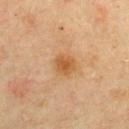Assessment: Part of a total-body skin-imaging series; this lesion was reviewed on a skin check and was not flagged for biopsy. Background: The total-body-photography lesion software estimated an area of roughly 5.5 mm², an eccentricity of roughly 0.4, and two-axis asymmetry of about 0.2. The software also gave an average lesion color of about L≈50 a*≈20 b*≈37 (CIELAB), about 9 CIELAB-L* units darker than the surrounding skin, and a normalized lesion–skin contrast near 7.5. The subject is a male approximately 60 years of age. This image is a 15 mm lesion crop taken from a total-body photograph. This is a cross-polarized tile. On the front of the torso. Longest diameter approximately 2.5 mm.